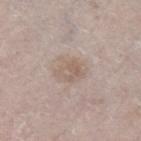No biopsy was performed on this lesion — it was imaged during a full skin examination and was not determined to be concerning.
The subject is a male about 65 years old.
The recorded lesion diameter is about 3.5 mm.
From the leg.
A 15 mm close-up extracted from a 3D total-body photography capture.